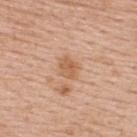The lesion was tiled from a total-body skin photograph and was not biopsied. This image is a 15 mm lesion crop taken from a total-body photograph. Captured under white-light illumination. From the upper back. The subject is a female aged approximately 65. The total-body-photography lesion software estimated a lesion area of about 5 mm², a shape eccentricity near 0.6, and a symmetry-axis asymmetry near 0.2. And it measured roughly 9 lightness units darker than nearby skin and a lesion-to-skin contrast of about 6.5 (normalized; higher = more distinct). And it measured a nevus-likeness score of about 5/100 and a lesion-detection confidence of about 100/100.